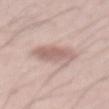The lesion's longest dimension is about 4 mm. On the abdomen. The tile uses white-light illumination. Automated tile analysis of the lesion measured an automated nevus-likeness rating near 50 out of 100. A male patient aged 53–57. A 15 mm crop from a total-body photograph taken for skin-cancer surveillance.